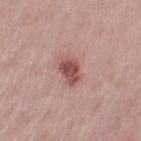automated lesion analysis = a nevus-likeness score of about 85/100 and a detector confidence of about 100 out of 100 that the crop contains a lesion | subject = female, aged approximately 40 | body site = the left upper arm | image = ~15 mm crop, total-body skin-cancer survey | size = about 3.5 mm | lighting = white-light illumination.